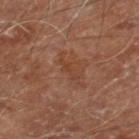| feature | finding |
|---|---|
| workup | no biopsy performed (imaged during a skin exam) |
| patient | male, about 70 years old |
| anatomic site | the right lower leg |
| lighting | cross-polarized |
| image source | 15 mm crop, total-body photography |